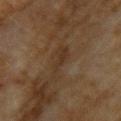Notes:
- biopsy status · imaged on a skin check; not biopsied
- illumination · cross-polarized illumination
- body site · the upper back
- image · ~15 mm tile from a whole-body skin photo
- patient · female, in their 60s
- image-analysis metrics · an area of roughly 5.5 mm² and a shape eccentricity near 0.95; a mean CIELAB color near L≈30 a*≈14 b*≈26, roughly 5 lightness units darker than nearby skin, and a normalized border contrast of about 5.5; a classifier nevus-likeness of about 0/100 and a lesion-detection confidence of about 80/100
- lesion size · ≈4.5 mm